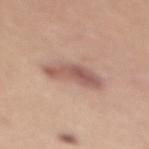Impression: Captured during whole-body skin photography for melanoma surveillance; the lesion was not biopsied. Clinical summary: Imaged with white-light lighting. The patient is a female aged 33–37. From the chest. The lesion's longest dimension is about 5.5 mm. Cropped from a total-body skin-imaging series; the visible field is about 15 mm.